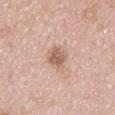Clinical impression:
Part of a total-body skin-imaging series; this lesion was reviewed on a skin check and was not flagged for biopsy.